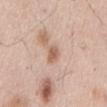The lesion was tiled from a total-body skin photograph and was not biopsied.
A lesion tile, about 15 mm wide, cut from a 3D total-body photograph.
From the mid back.
A male subject in their mid- to late 50s.
Longest diameter approximately 2.5 mm.
The tile uses white-light illumination.